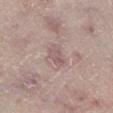Q: Was a biopsy performed?
A: catalogued during a skin exam; not biopsied
Q: What is the imaging modality?
A: ~15 mm crop, total-body skin-cancer survey
Q: What are the patient's age and sex?
A: male, roughly 65 years of age
Q: Lesion size?
A: ~2.5 mm (longest diameter)
Q: Lesion location?
A: the leg
Q: What did automated image analysis measure?
A: a lesion area of about 4 mm² and a shape eccentricity near 0.7; a mean CIELAB color near L≈56 a*≈18 b*≈18 and about 8 CIELAB-L* units darker than the surrounding skin; a border-irregularity index near 4/10 and radial color variation of about 1; a classifier nevus-likeness of about 0/100 and a lesion-detection confidence of about 95/100
Q: Illumination type?
A: white-light